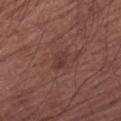{"biopsy_status": "not biopsied; imaged during a skin examination", "lighting": "white-light", "image": {"source": "total-body photography crop", "field_of_view_mm": 15}, "site": "left upper arm", "lesion_size": {"long_diameter_mm_approx": 2.5}, "patient": {"sex": "male", "age_approx": 65}}